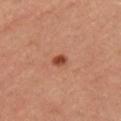Imaged during a routine full-body skin examination; the lesion was not biopsied and no histopathology is available. The lesion's longest dimension is about 2 mm. The subject is a male aged 63–67. Imaged with cross-polarized lighting. This image is a 15 mm lesion crop taken from a total-body photograph. The lesion-visualizer software estimated a footprint of about 2.5 mm², an eccentricity of roughly 0.55, and a shape-asymmetry score of about 0.25 (0 = symmetric). The software also gave a mean CIELAB color near L≈44 a*≈27 b*≈33 and about 12 CIELAB-L* units darker than the surrounding skin. And it measured a border-irregularity rating of about 2/10, a color-variation rating of about 2.5/10, and peripheral color asymmetry of about 1. The lesion is on the left upper arm.